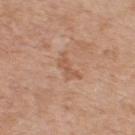Q: Was a biopsy performed?
A: imaged on a skin check; not biopsied
Q: What lighting was used for the tile?
A: white-light
Q: What is the imaging modality?
A: 15 mm crop, total-body photography
Q: Patient demographics?
A: male, approximately 55 years of age
Q: Where on the body is the lesion?
A: the upper back
Q: How large is the lesion?
A: about 3.5 mm